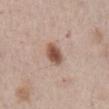Captured during whole-body skin photography for melanoma surveillance; the lesion was not biopsied. The subject is a male aged 63 to 67. This image is a 15 mm lesion crop taken from a total-body photograph. From the chest.